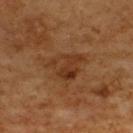{
  "biopsy_status": "not biopsied; imaged during a skin examination",
  "image": {
    "source": "total-body photography crop",
    "field_of_view_mm": 15
  },
  "site": "back",
  "patient": {
    "sex": "male",
    "age_approx": 60
  },
  "lesion_size": {
    "long_diameter_mm_approx": 4.0
  },
  "lighting": "cross-polarized"
}